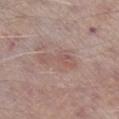{"biopsy_status": "not biopsied; imaged during a skin examination", "lesion_size": {"long_diameter_mm_approx": 5.0}, "lighting": "white-light", "patient": {"sex": "male", "age_approx": 65}, "automated_metrics": {"cielab_L": 54, "cielab_a": 19, "cielab_b": 22, "vs_skin_darker_L": 7.0, "vs_skin_contrast_norm": 5.0, "color_variation_0_10": 1.5, "peripheral_color_asymmetry": 0.5}, "image": {"source": "total-body photography crop", "field_of_view_mm": 15}, "site": "right lower leg"}